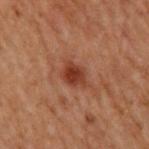<tbp_lesion>
<biopsy_status>not biopsied; imaged during a skin examination</biopsy_status>
<lesion_size>
  <long_diameter_mm_approx>2.5</long_diameter_mm_approx>
</lesion_size>
<lighting>cross-polarized</lighting>
<site>upper back</site>
<automated_metrics>
  <cielab_L>28</cielab_L>
  <cielab_a>22</cielab_a>
  <cielab_b>25</cielab_b>
  <vs_skin_contrast_norm>9.0</vs_skin_contrast_norm>
  <nevus_likeness_0_100>80</nevus_likeness_0_100>
  <lesion_detection_confidence_0_100>100</lesion_detection_confidence_0_100>
</automated_metrics>
<image>
  <source>total-body photography crop</source>
  <field_of_view_mm>15</field_of_view_mm>
</image>
<patient>
  <sex>male</sex>
  <age_approx>70</age_approx>
</patient>
</tbp_lesion>